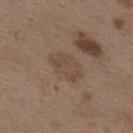No biopsy was performed on this lesion — it was imaged during a full skin examination and was not determined to be concerning. Captured under white-light illumination. A close-up tile cropped from a whole-body skin photograph, about 15 mm across. The lesion is on the upper back. The patient is a female in their mid- to late 30s.